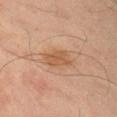Q: Is there a histopathology result?
A: total-body-photography surveillance lesion; no biopsy
Q: Illumination type?
A: cross-polarized
Q: Who is the patient?
A: male, aged around 65
Q: Lesion location?
A: the right upper arm
Q: What is the lesion's diameter?
A: ≈3 mm
Q: Automated lesion metrics?
A: a footprint of about 6.5 mm², an eccentricity of roughly 0.7, and a shape-asymmetry score of about 0.2 (0 = symmetric)
Q: What kind of image is this?
A: total-body-photography crop, ~15 mm field of view